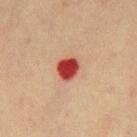Clinical impression: Recorded during total-body skin imaging; not selected for excision or biopsy. Acquisition and patient details: This image is a 15 mm lesion crop taken from a total-body photograph. The lesion's longest dimension is about 3 mm. Captured under cross-polarized illumination. On the chest. A male patient about 70 years old. The total-body-photography lesion software estimated a footprint of about 6 mm² and a shape-asymmetry score of about 0.15 (0 = symmetric). It also reported an average lesion color of about L≈38 a*≈34 b*≈27 (CIELAB), a lesion–skin lightness drop of about 17, and a lesion-to-skin contrast of about 13.5 (normalized; higher = more distinct).